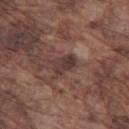Recorded during total-body skin imaging; not selected for excision or biopsy.
A region of skin cropped from a whole-body photographic capture, roughly 15 mm wide.
The tile uses white-light illumination.
The recorded lesion diameter is about 3 mm.
Automated image analysis of the tile measured a border-irregularity rating of about 3/10. The analysis additionally found a classifier nevus-likeness of about 0/100.
A male patient, in their mid-70s.
The lesion is on the left thigh.